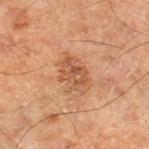Impression:
Captured during whole-body skin photography for melanoma surveillance; the lesion was not biopsied.
Context:
The lesion is on the right lower leg. A roughly 15 mm field-of-view crop from a total-body skin photograph. The subject is a male aged 63–67. The lesion-visualizer software estimated an eccentricity of roughly 0.75 and two-axis asymmetry of about 0.25. The software also gave a mean CIELAB color near L≈47 a*≈21 b*≈31, a lesion–skin lightness drop of about 8, and a normalized lesion–skin contrast near 6.5. The analysis additionally found a border-irregularity rating of about 3/10, a within-lesion color-variation index near 4.5/10, and peripheral color asymmetry of about 1.5. It also reported a nevus-likeness score of about 10/100.